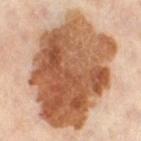Assessment:
Part of a total-body skin-imaging series; this lesion was reviewed on a skin check and was not flagged for biopsy.
Background:
A 15 mm close-up extracted from a 3D total-body photography capture. A female subject aged approximately 55. Approximately 13 mm at its widest. Located on the right thigh. The tile uses cross-polarized illumination.